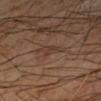notes=total-body-photography surveillance lesion; no biopsy | acquisition=~15 mm crop, total-body skin-cancer survey | location=the arm | illumination=cross-polarized illumination | subject=male, roughly 70 years of age | diameter=about 3 mm.